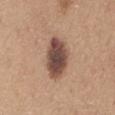| feature | finding |
|---|---|
| diameter | ≈6 mm |
| location | the mid back |
| subject | male, in their mid-60s |
| acquisition | ~15 mm tile from a whole-body skin photo |
| illumination | white-light |